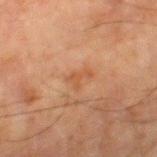Part of a total-body skin-imaging series; this lesion was reviewed on a skin check and was not flagged for biopsy. On the right thigh. Measured at roughly 3 mm in maximum diameter. This image is a 15 mm lesion crop taken from a total-body photograph. A male subject roughly 80 years of age. An algorithmic analysis of the crop reported a normalized border contrast of about 5. The analysis additionally found a classifier nevus-likeness of about 0/100 and a detector confidence of about 100 out of 100 that the crop contains a lesion.